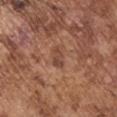Clinical impression: This lesion was catalogued during total-body skin photography and was not selected for biopsy. Context: Captured under white-light illumination. On the left upper arm. The patient is a male aged approximately 75. An algorithmic analysis of the crop reported a footprint of about 3 mm², an eccentricity of roughly 0.9, and two-axis asymmetry of about 0.55. The analysis additionally found a border-irregularity index near 5.5/10, internal color variation of about 0 on a 0–10 scale, and peripheral color asymmetry of about 0. The lesion's longest dimension is about 2.5 mm. Cropped from a total-body skin-imaging series; the visible field is about 15 mm.